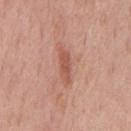Clinical impression: The lesion was tiled from a total-body skin photograph and was not biopsied.